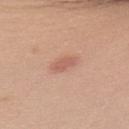Part of a total-body skin-imaging series; this lesion was reviewed on a skin check and was not flagged for biopsy. A close-up tile cropped from a whole-body skin photograph, about 15 mm across. A male subject, aged around 30.